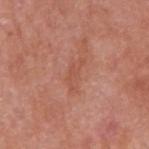Notes:
• follow-up — catalogued during a skin exam; not biopsied